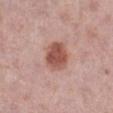Q: Was a biopsy performed?
A: no biopsy performed (imaged during a skin exam)